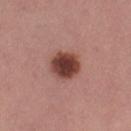Captured during whole-body skin photography for melanoma surveillance; the lesion was not biopsied.